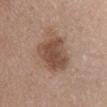follow-up: total-body-photography surveillance lesion; no biopsy
size: about 6.5 mm
automated lesion analysis: an outline eccentricity of about 0.85 (0 = round, 1 = elongated) and a symmetry-axis asymmetry near 0.25; an average lesion color of about L≈49 a*≈18 b*≈27 (CIELAB), about 12 CIELAB-L* units darker than the surrounding skin, and a normalized lesion–skin contrast near 8.5
location: the abdomen
tile lighting: white-light
imaging modality: 15 mm crop, total-body photography
subject: female, in their mid- to late 60s